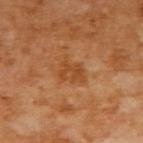Clinical impression:
The lesion was photographed on a routine skin check and not biopsied; there is no pathology result.
Clinical summary:
The lesion is located on the upper back. Imaged with cross-polarized lighting. The subject is a female aged 53 to 57. Automated image analysis of the tile measured a footprint of about 6 mm², a shape eccentricity near 0.65, and two-axis asymmetry of about 0.25. It also reported a lesion color around L≈48 a*≈26 b*≈41 in CIELAB and roughly 7 lightness units darker than nearby skin. The analysis additionally found a nevus-likeness score of about 5/100. A region of skin cropped from a whole-body photographic capture, roughly 15 mm wide.